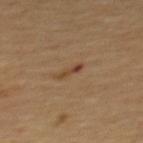Findings:
• biopsy status · no biopsy performed (imaged during a skin exam)
• image source · 15 mm crop, total-body photography
• body site · the mid back
• tile lighting · cross-polarized illumination
• lesion size · ≈2.5 mm
• subject · male, aged around 60